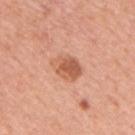Part of a total-body skin-imaging series; this lesion was reviewed on a skin check and was not flagged for biopsy.
Cropped from a total-body skin-imaging series; the visible field is about 15 mm.
Automated tile analysis of the lesion measured a mean CIELAB color near L≈59 a*≈26 b*≈34, a lesion–skin lightness drop of about 11, and a lesion-to-skin contrast of about 7.5 (normalized; higher = more distinct). The software also gave border irregularity of about 1.5 on a 0–10 scale, a color-variation rating of about 5/10, and a peripheral color-asymmetry measure near 1.5.
A male subject, in their mid-60s.
From the back.